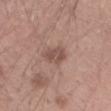biopsy_status: not biopsied; imaged during a skin examination
patient:
  sex: male
  age_approx: 45
automated_metrics:
  eccentricity: 0.7
  shape_asymmetry: 0.2
  border_irregularity_0_10: 2.5
  color_variation_0_10: 2.5
  peripheral_color_asymmetry: 1.0
  nevus_likeness_0_100: 10
  lesion_detection_confidence_0_100: 100
site: left forearm
lesion_size:
  long_diameter_mm_approx: 3.0
lighting: white-light
image:
  source: total-body photography crop
  field_of_view_mm: 15